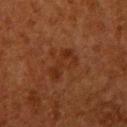Notes:
- follow-up · imaged on a skin check; not biopsied
- lighting · cross-polarized
- diameter · about 4 mm
- image source · 15 mm crop, total-body photography
- site · the right upper arm
- TBP lesion metrics · a lesion–skin lightness drop of about 5 and a lesion-to-skin contrast of about 5.5 (normalized; higher = more distinct); an automated nevus-likeness rating near 15 out of 100
- patient · female, aged 48–52